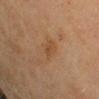<lesion>
<lighting>cross-polarized</lighting>
<lesion_size>
  <long_diameter_mm_approx>3.5</long_diameter_mm_approx>
</lesion_size>
<patient>
  <sex>female</sex>
  <age_approx>70</age_approx>
</patient>
<site>left upper arm</site>
<automated_metrics>
  <area_mm2_approx>4.0</area_mm2_approx>
  <eccentricity>0.9</eccentricity>
  <cielab_L>41</cielab_L>
  <cielab_a>17</cielab_a>
  <cielab_b>31</cielab_b>
  <vs_skin_darker_L>6.0</vs_skin_darker_L>
  <vs_skin_contrast_norm>5.5</vs_skin_contrast_norm>
  <nevus_likeness_0_100>0</nevus_likeness_0_100>
  <lesion_detection_confidence_0_100>100</lesion_detection_confidence_0_100>
</automated_metrics>
<image>
  <source>total-body photography crop</source>
  <field_of_view_mm>15</field_of_view_mm>
</image>
</lesion>